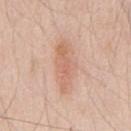Background:
This is a white-light tile. From the chest. Automated image analysis of the tile measured a nevus-likeness score of about 5/100 and lesion-presence confidence of about 100/100. About 6 mm across. A male subject, aged around 45. A region of skin cropped from a whole-body photographic capture, roughly 15 mm wide.